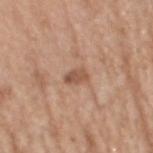Impression: Recorded during total-body skin imaging; not selected for excision or biopsy. Background: Captured under white-light illumination. A 15 mm close-up extracted from a 3D total-body photography capture. The subject is a male approximately 70 years of age. On the head or neck.